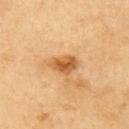Recorded during total-body skin imaging; not selected for excision or biopsy.
A 15 mm close-up tile from a total-body photography series done for melanoma screening.
The lesion-visualizer software estimated an area of roughly 6 mm² and an outline eccentricity of about 0.75 (0 = round, 1 = elongated). The analysis additionally found an average lesion color of about L≈58 a*≈23 b*≈43 (CIELAB), about 13 CIELAB-L* units darker than the surrounding skin, and a normalized lesion–skin contrast near 8.5. It also reported a border-irregularity rating of about 2/10, a color-variation rating of about 4/10, and a peripheral color-asymmetry measure near 1.5. The analysis additionally found a nevus-likeness score of about 85/100 and a lesion-detection confidence of about 100/100.
Approximately 3.5 mm at its widest.
A male patient, aged around 60.
On the arm.
Captured under cross-polarized illumination.